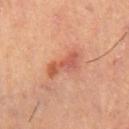Recorded during total-body skin imaging; not selected for excision or biopsy. A 15 mm close-up tile from a total-body photography series done for melanoma screening. A female patient, aged around 40. On the right thigh.